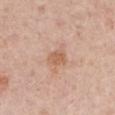<record>
  <biopsy_status>not biopsied; imaged during a skin examination</biopsy_status>
  <patient>
    <sex>male</sex>
    <age_approx>60</age_approx>
  </patient>
  <site>left upper arm</site>
  <image>
    <source>total-body photography crop</source>
    <field_of_view_mm>15</field_of_view_mm>
  </image>
</record>